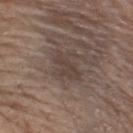{
  "biopsy_status": "not biopsied; imaged during a skin examination",
  "image": {
    "source": "total-body photography crop",
    "field_of_view_mm": 15
  },
  "patient": {
    "sex": "male",
    "age_approx": 75
  },
  "site": "front of the torso"
}